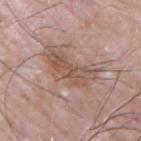Impression:
Imaged during a routine full-body skin examination; the lesion was not biopsied and no histopathology is available.
Context:
Longest diameter approximately 6 mm. A 15 mm close-up tile from a total-body photography series done for melanoma screening. The lesion is on the right upper arm. A male subject, about 65 years old. An algorithmic analysis of the crop reported a shape eccentricity near 0.9. And it measured about 9 CIELAB-L* units darker than the surrounding skin and a lesion-to-skin contrast of about 6.5 (normalized; higher = more distinct). The analysis additionally found internal color variation of about 4.5 on a 0–10 scale and a peripheral color-asymmetry measure near 1.5.